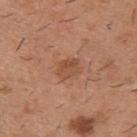The lesion was photographed on a routine skin check and not biopsied; there is no pathology result.
About 3 mm across.
A close-up tile cropped from a whole-body skin photograph, about 15 mm across.
The lesion-visualizer software estimated a shape eccentricity near 0.6. And it measured a lesion color around L≈50 a*≈24 b*≈32 in CIELAB, about 8 CIELAB-L* units darker than the surrounding skin, and a normalized border contrast of about 6. And it measured a detector confidence of about 100 out of 100 that the crop contains a lesion.
The subject is a male approximately 40 years of age.
This is a white-light tile.
The lesion is located on the upper back.